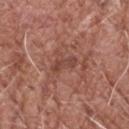Assessment: Recorded during total-body skin imaging; not selected for excision or biopsy. Acquisition and patient details: The total-body-photography lesion software estimated a lesion area of about 3.5 mm², an outline eccentricity of about 0.9 (0 = round, 1 = elongated), and two-axis asymmetry of about 0.35. A male subject, aged 68–72. This is a white-light tile. The lesion is on the head or neck. A close-up tile cropped from a whole-body skin photograph, about 15 mm across. Approximately 3 mm at its widest.